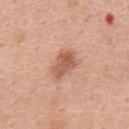{
  "lesion_size": {
    "long_diameter_mm_approx": 4.0
  },
  "image": {
    "source": "total-body photography crop",
    "field_of_view_mm": 15
  },
  "site": "back",
  "patient": {
    "sex": "male",
    "age_approx": 70
  }
}